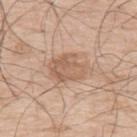| feature | finding |
|---|---|
| notes | catalogued during a skin exam; not biopsied |
| site | the upper back |
| illumination | white-light illumination |
| acquisition | ~15 mm tile from a whole-body skin photo |
| diameter | ≈5.5 mm |
| subject | male, in their 70s |